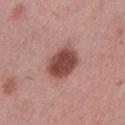A roughly 15 mm field-of-view crop from a total-body skin photograph.
On the left lower leg.
The patient is a female aged around 50.